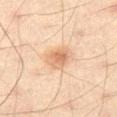* illumination: cross-polarized illumination
* lesion diameter: ~3.5 mm (longest diameter)
* patient: male, roughly 40 years of age
* image: ~15 mm crop, total-body skin-cancer survey
* anatomic site: the left thigh
* automated lesion analysis: a within-lesion color-variation index near 4/10 and a peripheral color-asymmetry measure near 1.5; an automated nevus-likeness rating near 85 out of 100 and a detector confidence of about 100 out of 100 that the crop contains a lesion